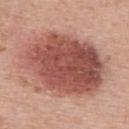Histopathology of the biopsied lesion showed a melanoma in situ arising in association with a nevus.